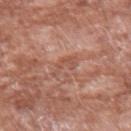Imaged during a routine full-body skin examination; the lesion was not biopsied and no histopathology is available. An algorithmic analysis of the crop reported a footprint of about 4 mm² and a shape-asymmetry score of about 0.5 (0 = symmetric). The analysis additionally found peripheral color asymmetry of about 0.5. The software also gave a detector confidence of about 90 out of 100 that the crop contains a lesion. A close-up tile cropped from a whole-body skin photograph, about 15 mm across. On the left forearm. Approximately 3 mm at its widest. The tile uses white-light illumination. The subject is a male aged 58 to 62.